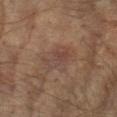This lesion was catalogued during total-body skin photography and was not selected for biopsy.
A male patient about 65 years old.
Located on the left forearm.
Cropped from a whole-body photographic skin survey; the tile spans about 15 mm.